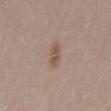• notes: total-body-photography surveillance lesion; no biopsy
• acquisition: ~15 mm tile from a whole-body skin photo
• automated lesion analysis: an area of roughly 4 mm² and an eccentricity of roughly 0.85; a color-variation rating of about 2/10 and a peripheral color-asymmetry measure near 0.5; an automated nevus-likeness rating near 35 out of 100 and a detector confidence of about 100 out of 100 that the crop contains a lesion
• lesion size: about 3 mm
• location: the mid back
• subject: male, about 30 years old
• illumination: white-light illumination